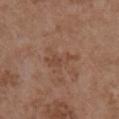Clinical impression: Captured during whole-body skin photography for melanoma surveillance; the lesion was not biopsied. Background: A male subject aged approximately 55. This is a white-light tile. Cropped from a total-body skin-imaging series; the visible field is about 15 mm. The lesion is located on the right upper arm.